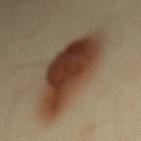Assessment:
The lesion was tiled from a total-body skin photograph and was not biopsied.
Image and clinical context:
This image is a 15 mm lesion crop taken from a total-body photograph. On the mid back. The subject is a female aged 43 to 47. This is a cross-polarized tile.